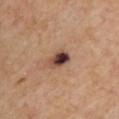Captured during whole-body skin photography for melanoma surveillance; the lesion was not biopsied.
Approximately 3 mm at its widest.
The patient is a male about 70 years old.
This is a cross-polarized tile.
Cropped from a whole-body photographic skin survey; the tile spans about 15 mm.
On the chest.